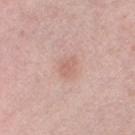A 15 mm close-up extracted from a 3D total-body photography capture.
A male subject about 60 years old.
From the right thigh.
The lesion's longest dimension is about 2.5 mm.
This is a white-light tile.
Automated tile analysis of the lesion measured a lesion area of about 3.5 mm², a shape eccentricity near 0.7, and a symmetry-axis asymmetry near 0.35. And it measured a border-irregularity rating of about 3/10 and a color-variation rating of about 2/10.